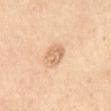Clinical impression: Captured during whole-body skin photography for melanoma surveillance; the lesion was not biopsied. Background: A female patient about 45 years old. The lesion is located on the abdomen. A 15 mm close-up extracted from a 3D total-body photography capture.